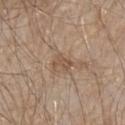Impression:
Imaged during a routine full-body skin examination; the lesion was not biopsied and no histopathology is available.
Clinical summary:
The subject is a male about 65 years old. Cropped from a whole-body photographic skin survey; the tile spans about 15 mm. Imaged with white-light lighting. The lesion is on the arm. Longest diameter approximately 2.5 mm.